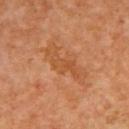Context:
Longest diameter approximately 5 mm. An algorithmic analysis of the crop reported a lesion area of about 6 mm², an outline eccentricity of about 0.95 (0 = round, 1 = elongated), and a symmetry-axis asymmetry near 0.45. And it measured an average lesion color of about L≈52 a*≈27 b*≈40 (CIELAB) and a normalized lesion–skin contrast near 5.5. It also reported a nevus-likeness score of about 0/100 and a lesion-detection confidence of about 100/100. Imaged with cross-polarized lighting. A female subject, aged 38–42. A 15 mm crop from a total-body photograph taken for skin-cancer surveillance. From the upper back.